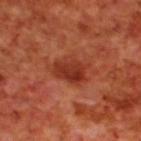Part of a total-body skin-imaging series; this lesion was reviewed on a skin check and was not flagged for biopsy. The lesion is located on the upper back. Approximately 4 mm at its widest. A close-up tile cropped from a whole-body skin photograph, about 15 mm across. Automated tile analysis of the lesion measured a footprint of about 9.5 mm², an eccentricity of roughly 0.7, and two-axis asymmetry of about 0.25. The analysis additionally found an average lesion color of about L≈35 a*≈31 b*≈33 (CIELAB) and a lesion-to-skin contrast of about 8 (normalized; higher = more distinct). The subject is a male aged around 70.